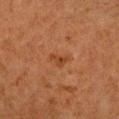• imaging modality — 15 mm crop, total-body photography
• illumination — cross-polarized illumination
• anatomic site — the arm
• patient — female, aged approximately 40